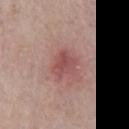No biopsy was performed on this lesion — it was imaged during a full skin examination and was not determined to be concerning.
A close-up tile cropped from a whole-body skin photograph, about 15 mm across.
On the front of the torso.
A male patient, aged 58 to 62.